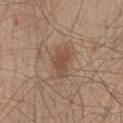This lesion was catalogued during total-body skin photography and was not selected for biopsy. The subject is a male aged 43–47. A 15 mm crop from a total-body photograph taken for skin-cancer surveillance. The tile uses white-light illumination. Automated tile analysis of the lesion measured a footprint of about 7.5 mm², an outline eccentricity of about 0.8 (0 = round, 1 = elongated), and a symmetry-axis asymmetry near 0.3. It also reported a border-irregularity rating of about 3.5/10, a within-lesion color-variation index near 3.5/10, and a peripheral color-asymmetry measure near 1. The analysis additionally found a nevus-likeness score of about 45/100. Longest diameter approximately 4 mm. The lesion is on the mid back.